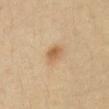workup = no biopsy performed (imaged during a skin exam)
image source = ~15 mm tile from a whole-body skin photo
location = the abdomen
illumination = cross-polarized
subject = female, in their mid-30s